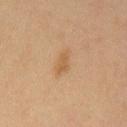Assessment:
Captured during whole-body skin photography for melanoma surveillance; the lesion was not biopsied.
Background:
Longest diameter approximately 3.5 mm. A male patient aged 43–47. A region of skin cropped from a whole-body photographic capture, roughly 15 mm wide. Captured under cross-polarized illumination. On the chest.